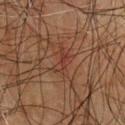{"biopsy_status": "not biopsied; imaged during a skin examination", "lighting": "cross-polarized", "patient": {"sex": "male", "age_approx": 65}, "automated_metrics": {"area_mm2_approx": 3.5, "eccentricity": 0.85, "shape_asymmetry": 0.4, "border_irregularity_0_10": 4.5, "color_variation_0_10": 5.0, "peripheral_color_asymmetry": 2.0, "nevus_likeness_0_100": 0, "lesion_detection_confidence_0_100": 90}, "image": {"source": "total-body photography crop", "field_of_view_mm": 15}, "site": "chest", "lesion_size": {"long_diameter_mm_approx": 3.0}}